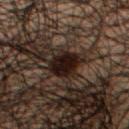| feature | finding |
|---|---|
| workup | imaged on a skin check; not biopsied |
| lighting | cross-polarized |
| location | the mid back |
| size | ~4 mm (longest diameter) |
| patient | male, aged 48–52 |
| image source | ~15 mm crop, total-body skin-cancer survey |